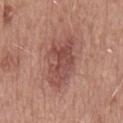biopsy_status: not biopsied; imaged during a skin examination
patient:
  sex: male
  age_approx: 50
automated_metrics:
  area_mm2_approx: 19.0
  eccentricity: 0.85
  shape_asymmetry: 0.25
  border_irregularity_0_10: 3.5
  color_variation_0_10: 4.5
  peripheral_color_asymmetry: 1.5
image:
  source: total-body photography crop
  field_of_view_mm: 15
site: mid back
lesion_size:
  long_diameter_mm_approx: 7.0